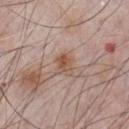The lesion was tiled from a total-body skin photograph and was not biopsied. A 15 mm crop from a total-body photograph taken for skin-cancer surveillance. The lesion's longest dimension is about 2.5 mm. The lesion is located on the chest. A male subject, in their mid-60s.